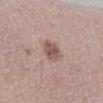<lesion>
<biopsy_status>not biopsied; imaged during a skin examination</biopsy_status>
<patient>
  <sex>female</sex>
  <age_approx>50</age_approx>
</patient>
<lighting>white-light</lighting>
<site>left lower leg</site>
<image>
  <source>total-body photography crop</source>
  <field_of_view_mm>15</field_of_view_mm>
</image>
<lesion_size>
  <long_diameter_mm_approx>3.0</long_diameter_mm_approx>
</lesion_size>
</lesion>